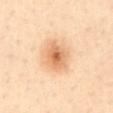{"image": {"source": "total-body photography crop", "field_of_view_mm": 15}, "patient": {"sex": "female", "age_approx": 45}, "site": "front of the torso"}